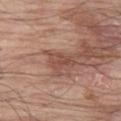follow-up — no biopsy performed (imaged during a skin exam) | tile lighting — white-light illumination | diameter — about 3.5 mm | image — ~15 mm tile from a whole-body skin photo | subject — male, aged 78–82 | site — the left thigh.